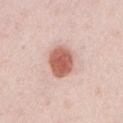Context:
About 4 mm across. The subject is a male in their mid- to late 20s. Captured under white-light illumination. A 15 mm close-up tile from a total-body photography series done for melanoma screening. From the upper back. The lesion-visualizer software estimated a footprint of about 10 mm², a shape eccentricity near 0.65, and a shape-asymmetry score of about 0.15 (0 = symmetric).